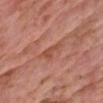Recorded during total-body skin imaging; not selected for excision or biopsy. Automated tile analysis of the lesion measured border irregularity of about 4.5 on a 0–10 scale, internal color variation of about 2.5 on a 0–10 scale, and peripheral color asymmetry of about 0.5. The software also gave a classifier nevus-likeness of about 0/100. The tile uses white-light illumination. On the chest. A roughly 15 mm field-of-view crop from a total-body skin photograph. A male patient aged around 60. Longest diameter approximately 4 mm.